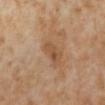Part of a total-body skin-imaging series; this lesion was reviewed on a skin check and was not flagged for biopsy. The subject is a female approximately 50 years of age. The lesion is on the left lower leg. This image is a 15 mm lesion crop taken from a total-body photograph.